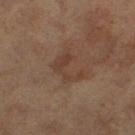biopsy_status: not biopsied; imaged during a skin examination
lesion_size:
  long_diameter_mm_approx: 4.0
site: left thigh
patient:
  sex: female
  age_approx: 60
image:
  source: total-body photography crop
  field_of_view_mm: 15
automated_metrics:
  area_mm2_approx: 7.5
  eccentricity: 0.75
  shape_asymmetry: 0.5
  cielab_L: 34
  cielab_a: 15
  cielab_b: 23
  vs_skin_darker_L: 5.0
  vs_skin_contrast_norm: 5.5
  border_irregularity_0_10: 8.0
  color_variation_0_10: 1.5
  peripheral_color_asymmetry: 0.5